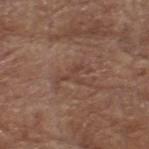biopsy_status: not biopsied; imaged during a skin examination
site: head or neck
patient:
  sex: male
  age_approx: 80
automated_metrics:
  cielab_L: 42
  cielab_a: 19
  cielab_b: 25
  vs_skin_contrast_norm: 5.0
  border_irregularity_0_10: 7.0
  color_variation_0_10: 0.0
  nevus_likeness_0_100: 0
  lesion_detection_confidence_0_100: 90
lighting: white-light
image:
  source: total-body photography crop
  field_of_view_mm: 15
lesion_size:
  long_diameter_mm_approx: 3.5